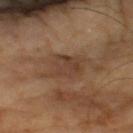{
  "biopsy_status": "not biopsied; imaged during a skin examination",
  "image": {
    "source": "total-body photography crop",
    "field_of_view_mm": 15
  },
  "patient": {
    "sex": "male",
    "age_approx": 65
  }
}